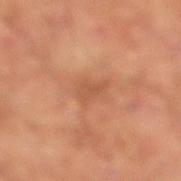The lesion was photographed on a routine skin check and not biopsied; there is no pathology result. The subject is aged 53 to 57. Automated tile analysis of the lesion measured an outline eccentricity of about 0.9 (0 = round, 1 = elongated) and two-axis asymmetry of about 0.4. The software also gave a mean CIELAB color near L≈53 a*≈25 b*≈35, about 7 CIELAB-L* units darker than the surrounding skin, and a lesion-to-skin contrast of about 5 (normalized; higher = more distinct). It also reported border irregularity of about 4 on a 0–10 scale, a within-lesion color-variation index near 0.5/10, and radial color variation of about 0. The software also gave a nevus-likeness score of about 0/100 and a lesion-detection confidence of about 100/100. On the leg. This is a cross-polarized tile. A 15 mm close-up extracted from a 3D total-body photography capture. Approximately 3 mm at its widest.